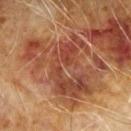{
  "biopsy_status": "not biopsied; imaged during a skin examination",
  "patient": {
    "sex": "male",
    "age_approx": 70
  },
  "lighting": "cross-polarized",
  "lesion_size": {
    "long_diameter_mm_approx": 10.0
  },
  "image": {
    "source": "total-body photography crop",
    "field_of_view_mm": 15
  },
  "site": "arm",
  "automated_metrics": {
    "area_mm2_approx": 45.0,
    "eccentricity": 0.75,
    "shape_asymmetry": 0.45,
    "nevus_likeness_0_100": 0,
    "lesion_detection_confidence_0_100": 65
  }
}